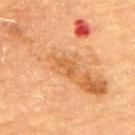Part of a total-body skin-imaging series; this lesion was reviewed on a skin check and was not flagged for biopsy.
An algorithmic analysis of the crop reported a lesion area of about 3.5 mm², a shape eccentricity near 0.85, and a symmetry-axis asymmetry near 0.4. The software also gave a border-irregularity index near 4.5/10 and a color-variation rating of about 1.5/10. The analysis additionally found a classifier nevus-likeness of about 0/100 and a detector confidence of about 100 out of 100 that the crop contains a lesion.
Located on the upper back.
Approximately 3 mm at its widest.
This is a cross-polarized tile.
A female subject approximately 70 years of age.
A 15 mm crop from a total-body photograph taken for skin-cancer surveillance.